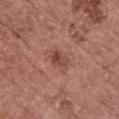Q: Was a biopsy performed?
A: catalogued during a skin exam; not biopsied
Q: What lighting was used for the tile?
A: white-light
Q: Patient demographics?
A: female, roughly 65 years of age
Q: What kind of image is this?
A: 15 mm crop, total-body photography
Q: Lesion size?
A: ≈2.5 mm
Q: What is the anatomic site?
A: the chest
Q: Automated lesion metrics?
A: a classifier nevus-likeness of about 5/100 and a detector confidence of about 100 out of 100 that the crop contains a lesion